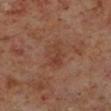  biopsy_status: not biopsied; imaged during a skin examination
  lesion_size:
    long_diameter_mm_approx: 4.0
  lighting: cross-polarized
  patient:
    sex: male
    age_approx: 60
  image:
    source: total-body photography crop
    field_of_view_mm: 15
  site: left lower leg